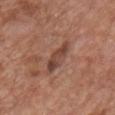<record>
<biopsy_status>not biopsied; imaged during a skin examination</biopsy_status>
<site>chest</site>
<automated_metrics>
  <border_irregularity_0_10>4.0</border_irregularity_0_10>
  <color_variation_0_10>3.0</color_variation_0_10>
  <peripheral_color_asymmetry>0.5</peripheral_color_asymmetry>
  <nevus_likeness_0_100>0</nevus_likeness_0_100>
  <lesion_detection_confidence_0_100>100</lesion_detection_confidence_0_100>
</automated_metrics>
<image>
  <source>total-body photography crop</source>
  <field_of_view_mm>15</field_of_view_mm>
</image>
<patient>
  <sex>male</sex>
  <age_approx>65</age_approx>
</patient>
<lesion_size>
  <long_diameter_mm_approx>4.5</long_diameter_mm_approx>
</lesion_size>
</record>